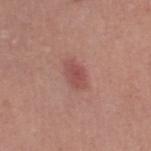  image:
    source: total-body photography crop
    field_of_view_mm: 15
  patient:
    sex: female
    age_approx: 45
  site: right thigh